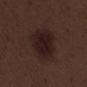follow-up — catalogued during a skin exam; not biopsied | automated metrics — a lesion-to-skin contrast of about 10.5 (normalized; higher = more distinct); lesion-presence confidence of about 100/100 | body site — the left thigh | subject — male, aged approximately 70 | tile lighting — white-light | image — ~15 mm tile from a whole-body skin photo.